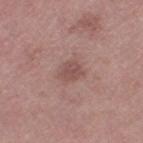<tbp_lesion>
  <biopsy_status>not biopsied; imaged during a skin examination</biopsy_status>
  <automated_metrics>
    <border_irregularity_0_10>3.0</border_irregularity_0_10>
  </automated_metrics>
  <image>
    <source>total-body photography crop</source>
    <field_of_view_mm>15</field_of_view_mm>
  </image>
  <site>left thigh</site>
  <lesion_size>
    <long_diameter_mm_approx>2.5</long_diameter_mm_approx>
  </lesion_size>
  <lighting>white-light</lighting>
  <patient>
    <sex>female</sex>
    <age_approx>70</age_approx>
  </patient>
</tbp_lesion>